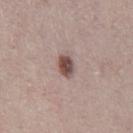– biopsy status — no biopsy performed (imaged during a skin exam)
– image source — 15 mm crop, total-body photography
– anatomic site — the right thigh
– patient — female, aged 38 to 42
– lighting — white-light illumination
– image-analysis metrics — a footprint of about 4 mm², an eccentricity of roughly 0.7, and two-axis asymmetry of about 0.3; an automated nevus-likeness rating near 95 out of 100 and a lesion-detection confidence of about 100/100